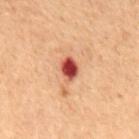Clinical impression: Captured during whole-body skin photography for melanoma surveillance; the lesion was not biopsied. Clinical summary: A female subject aged approximately 80. The lesion is located on the chest. The tile uses cross-polarized illumination. A region of skin cropped from a whole-body photographic capture, roughly 15 mm wide. The recorded lesion diameter is about 2.5 mm.